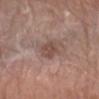Findings:
– follow-up · total-body-photography surveillance lesion; no biopsy
– image · ~15 mm crop, total-body skin-cancer survey
– illumination · white-light illumination
– location · the left forearm
– lesion diameter · about 3.5 mm
– subject · male, approximately 80 years of age